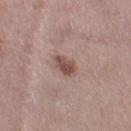Assessment:
The lesion was tiled from a total-body skin photograph and was not biopsied.
Background:
The lesion is on the right thigh. A 15 mm crop from a total-body photograph taken for skin-cancer surveillance. A female patient in their 40s.